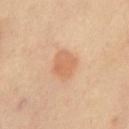workup: no biopsy performed (imaged during a skin exam)
diameter: ~3 mm (longest diameter)
image source: total-body-photography crop, ~15 mm field of view
illumination: cross-polarized
body site: the chest